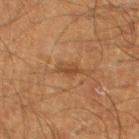{"biopsy_status": "not biopsied; imaged during a skin examination", "patient": {"sex": "male", "age_approx": 50}, "lighting": "cross-polarized", "lesion_size": {"long_diameter_mm_approx": 2.5}, "image": {"source": "total-body photography crop", "field_of_view_mm": 15}, "automated_metrics": {"area_mm2_approx": 3.0, "cielab_L": 35, "cielab_a": 17, "cielab_b": 29, "vs_skin_darker_L": 6.0, "nevus_likeness_0_100": 5, "lesion_detection_confidence_0_100": 100}, "site": "left lower leg"}